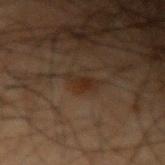On the left forearm. The subject is a male roughly 50 years of age. The total-body-photography lesion software estimated an area of roughly 4 mm², an outline eccentricity of about 0.8 (0 = round, 1 = elongated), and a symmetry-axis asymmetry near 0.35. The analysis additionally found border irregularity of about 3.5 on a 0–10 scale, a color-variation rating of about 1/10, and peripheral color asymmetry of about 0.5. It also reported an automated nevus-likeness rating near 35 out of 100 and a lesion-detection confidence of about 100/100. Measured at roughly 3 mm in maximum diameter. Cropped from a total-body skin-imaging series; the visible field is about 15 mm.